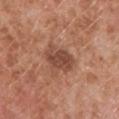Impression: Imaged during a routine full-body skin examination; the lesion was not biopsied and no histopathology is available. Context: Measured at roughly 4.5 mm in maximum diameter. Located on the left lower leg. Captured under white-light illumination. A male patient aged around 80. This image is a 15 mm lesion crop taken from a total-body photograph.